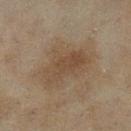• notes: total-body-photography surveillance lesion; no biopsy
• image: ~15 mm crop, total-body skin-cancer survey
• location: the leg
• TBP lesion metrics: an average lesion color of about L≈42 a*≈13 b*≈27 (CIELAB), about 7 CIELAB-L* units darker than the surrounding skin, and a normalized lesion–skin contrast near 6; a peripheral color-asymmetry measure near 1
• patient: female, about 60 years old
• lighting: cross-polarized